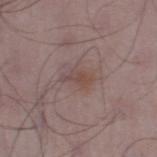Part of a total-body skin-imaging series; this lesion was reviewed on a skin check and was not flagged for biopsy.
Measured at roughly 3 mm in maximum diameter.
Captured under white-light illumination.
The lesion is on the left lower leg.
A male subject aged 48–52.
A roughly 15 mm field-of-view crop from a total-body skin photograph.